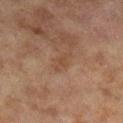Q: Was this lesion biopsied?
A: total-body-photography surveillance lesion; no biopsy
Q: Where on the body is the lesion?
A: the right lower leg
Q: Illumination type?
A: cross-polarized illumination
Q: What are the patient's age and sex?
A: female, approximately 60 years of age
Q: What did automated image analysis measure?
A: a border-irregularity rating of about 3.5/10, internal color variation of about 1 on a 0–10 scale, and radial color variation of about 0
Q: What is the imaging modality?
A: ~15 mm tile from a whole-body skin photo
Q: Lesion size?
A: ~3 mm (longest diameter)